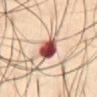This lesion was catalogued during total-body skin photography and was not selected for biopsy.
Imaged with cross-polarized lighting.
A 15 mm close-up tile from a total-body photography series done for melanoma screening.
Approximately 3.5 mm at its widest.
A male patient, approximately 50 years of age.
The lesion is on the front of the torso.